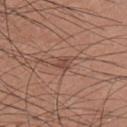notes: catalogued during a skin exam; not biopsied | tile lighting: white-light illumination | imaging modality: 15 mm crop, total-body photography | location: the left upper arm | patient: male, about 35 years old | lesion diameter: ≈3 mm.